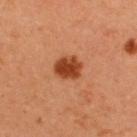{"biopsy_status": "not biopsied; imaged during a skin examination", "lighting": "cross-polarized", "automated_metrics": {"border_irregularity_0_10": 1.5, "color_variation_0_10": 3.0, "peripheral_color_asymmetry": 1.0}, "site": "upper back", "patient": {"sex": "male", "age_approx": 35}, "image": {"source": "total-body photography crop", "field_of_view_mm": 15}, "lesion_size": {"long_diameter_mm_approx": 3.0}}